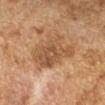{
  "biopsy_status": "not biopsied; imaged during a skin examination",
  "lighting": "cross-polarized",
  "image": {
    "source": "total-body photography crop",
    "field_of_view_mm": 15
  },
  "automated_metrics": {
    "cielab_L": 45,
    "cielab_a": 18,
    "cielab_b": 31,
    "vs_skin_darker_L": 8.0,
    "nevus_likeness_0_100": 10,
    "lesion_detection_confidence_0_100": 100
  },
  "patient": {
    "sex": "female",
    "age_approx": 60
  },
  "site": "left forearm",
  "lesion_size": {
    "long_diameter_mm_approx": 5.0
  }
}